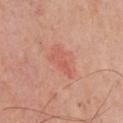| key | value |
|---|---|
| workup | catalogued during a skin exam; not biopsied |